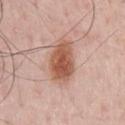This lesion was catalogued during total-body skin photography and was not selected for biopsy.
Cropped from a total-body skin-imaging series; the visible field is about 15 mm.
This is a white-light tile.
Automated image analysis of the tile measured an area of roughly 14 mm², an outline eccentricity of about 0.85 (0 = round, 1 = elongated), and a shape-asymmetry score of about 0.15 (0 = symmetric). The software also gave about 13 CIELAB-L* units darker than the surrounding skin and a normalized border contrast of about 9.5. It also reported a within-lesion color-variation index near 4.5/10 and radial color variation of about 1.5. The software also gave a classifier nevus-likeness of about 100/100 and lesion-presence confidence of about 100/100.
The recorded lesion diameter is about 5.5 mm.
The lesion is on the front of the torso.
A male subject, in their 60s.